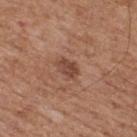Imaged during a routine full-body skin examination; the lesion was not biopsied and no histopathology is available.
From the upper back.
Measured at roughly 3 mm in maximum diameter.
A region of skin cropped from a whole-body photographic capture, roughly 15 mm wide.
A male patient aged around 65.
The tile uses white-light illumination.